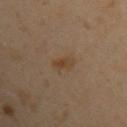{
  "biopsy_status": "not biopsied; imaged during a skin examination",
  "automated_metrics": {
    "cielab_L": 40,
    "cielab_a": 15,
    "cielab_b": 29,
    "vs_skin_darker_L": 6.0,
    "vs_skin_contrast_norm": 6.5,
    "color_variation_0_10": 3.0,
    "peripheral_color_asymmetry": 1.0
  },
  "image": {
    "source": "total-body photography crop",
    "field_of_view_mm": 15
  },
  "lighting": "cross-polarized",
  "lesion_size": {
    "long_diameter_mm_approx": 3.0
  },
  "patient": {
    "sex": "male",
    "age_approx": 40
  },
  "site": "left upper arm"
}